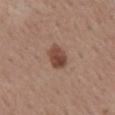No biopsy was performed on this lesion — it was imaged during a full skin examination and was not determined to be concerning.
The lesion is on the mid back.
Longest diameter approximately 3 mm.
Imaged with white-light lighting.
A 15 mm close-up extracted from a 3D total-body photography capture.
The patient is a male roughly 55 years of age.